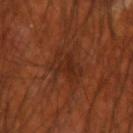– biopsy status: imaged on a skin check; not biopsied
– patient: male, aged 68 to 72
– acquisition: ~15 mm crop, total-body skin-cancer survey
– location: the right upper arm